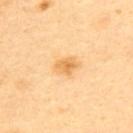Recorded during total-body skin imaging; not selected for excision or biopsy.
From the upper back.
Captured under cross-polarized illumination.
A male patient, in their 40s.
The total-body-photography lesion software estimated a lesion area of about 4.5 mm², an eccentricity of roughly 0.7, and two-axis asymmetry of about 0.2. The software also gave roughly 10 lightness units darker than nearby skin and a normalized border contrast of about 7.
A roughly 15 mm field-of-view crop from a total-body skin photograph.
Measured at roughly 3 mm in maximum diameter.